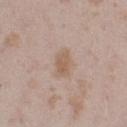follow-up = imaged on a skin check; not biopsied
anatomic site = the left thigh
diameter = ~3.5 mm (longest diameter)
image = 15 mm crop, total-body photography
subject = female, in their mid-20s
TBP lesion metrics = a footprint of about 6 mm² and a symmetry-axis asymmetry near 0.25; a mean CIELAB color near L≈59 a*≈16 b*≈28, roughly 8 lightness units darker than nearby skin, and a normalized lesion–skin contrast near 6.5; a border-irregularity rating of about 2.5/10 and a peripheral color-asymmetry measure near 1
tile lighting = white-light